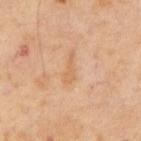The lesion was tiled from a total-body skin photograph and was not biopsied. A roughly 15 mm field-of-view crop from a total-body skin photograph. A male subject, aged 63 to 67. Longest diameter approximately 3.5 mm. Imaged with cross-polarized lighting.